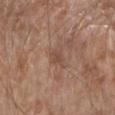Clinical impression:
The lesion was photographed on a routine skin check and not biopsied; there is no pathology result.